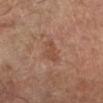Assessment: Recorded during total-body skin imaging; not selected for excision or biopsy. Context: From the left lower leg. An algorithmic analysis of the crop reported a shape eccentricity near 0.75 and two-axis asymmetry of about 0.35. The analysis additionally found a mean CIELAB color near L≈47 a*≈21 b*≈29, a lesion–skin lightness drop of about 6, and a normalized lesion–skin contrast near 5. The software also gave a border-irregularity index near 3.5/10, a within-lesion color-variation index near 1.5/10, and peripheral color asymmetry of about 0.5. A patient about 65 years old. The tile uses cross-polarized illumination. Approximately 3 mm at its widest. A 15 mm crop from a total-body photograph taken for skin-cancer surveillance.